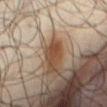Impression:
Recorded during total-body skin imaging; not selected for excision or biopsy.
Acquisition and patient details:
Imaged with cross-polarized lighting. The lesion-visualizer software estimated two-axis asymmetry of about 0.3. From the abdomen. This image is a 15 mm lesion crop taken from a total-body photograph. A male patient, aged approximately 65. Measured at roughly 3.5 mm in maximum diameter.